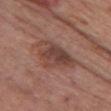Findings:
• notes · total-body-photography surveillance lesion; no biopsy
• lesion size · ~6 mm (longest diameter)
• imaging modality · total-body-photography crop, ~15 mm field of view
• location · the chest
• patient · female, aged around 60
• lighting · white-light illumination